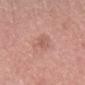<record>
  <site>head or neck</site>
  <automated_metrics>
    <cielab_L>57</cielab_L>
    <cielab_a>23</cielab_a>
    <cielab_b>27</cielab_b>
    <nevus_likeness_0_100>0</nevus_likeness_0_100>
    <lesion_detection_confidence_0_100>100</lesion_detection_confidence_0_100>
  </automated_metrics>
  <lesion_size>
    <long_diameter_mm_approx>2.5</long_diameter_mm_approx>
  </lesion_size>
  <image>
    <source>total-body photography crop</source>
    <field_of_view_mm>15</field_of_view_mm>
  </image>
  <lighting>white-light</lighting>
  <patient>
    <sex>female</sex>
    <age_approx>60</age_approx>
  </patient>
</record>